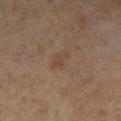Clinical impression: The lesion was tiled from a total-body skin photograph and was not biopsied. Acquisition and patient details: A region of skin cropped from a whole-body photographic capture, roughly 15 mm wide. The lesion is on the right lower leg. A male subject about 65 years old. About 3 mm across. This is a cross-polarized tile.